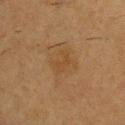Imaged during a routine full-body skin examination; the lesion was not biopsied and no histopathology is available.
The lesion's longest dimension is about 3 mm.
The lesion is located on the left upper arm.
A 15 mm close-up extracted from a 3D total-body photography capture.
The tile uses cross-polarized illumination.
A male patient, approximately 55 years of age.
The total-body-photography lesion software estimated a footprint of about 5 mm², an eccentricity of roughly 0.75, and a symmetry-axis asymmetry near 0.3. And it measured a classifier nevus-likeness of about 10/100 and a detector confidence of about 100 out of 100 that the crop contains a lesion.